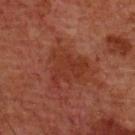| key | value |
|---|---|
| biopsy status | total-body-photography surveillance lesion; no biopsy |
| body site | the chest |
| acquisition | 15 mm crop, total-body photography |
| lesion size | about 5 mm |
| patient | male, aged 58–62 |